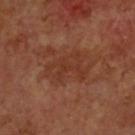image: 15 mm crop, total-body photography
tile lighting: cross-polarized
diameter: ~5 mm (longest diameter)
location: the head or neck
subject: male, approximately 60 years of age